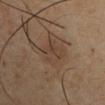A male subject in their 40s. Located on the left upper arm. Cropped from a total-body skin-imaging series; the visible field is about 15 mm.